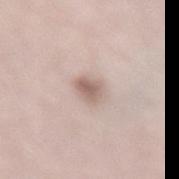The lesion was photographed on a routine skin check and not biopsied; there is no pathology result. The lesion is on the lower back. The patient is a female roughly 50 years of age. Cropped from a total-body skin-imaging series; the visible field is about 15 mm.